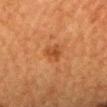Part of a total-body skin-imaging series; this lesion was reviewed on a skin check and was not flagged for biopsy. A region of skin cropped from a whole-body photographic capture, roughly 15 mm wide. The lesion is on the head or neck. The patient is a female roughly 50 years of age. The total-body-photography lesion software estimated a footprint of about 4.5 mm², an eccentricity of roughly 0.5, and a symmetry-axis asymmetry near 0.3. The software also gave a mean CIELAB color near L≈40 a*≈23 b*≈35 and a lesion–skin lightness drop of about 7. It also reported a classifier nevus-likeness of about 25/100 and lesion-presence confidence of about 100/100. The recorded lesion diameter is about 2.5 mm. This is a cross-polarized tile.